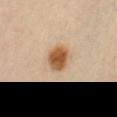Q: What did automated image analysis measure?
A: a footprint of about 7.5 mm² and a shape-asymmetry score of about 0.1 (0 = symmetric); an automated nevus-likeness rating near 100 out of 100 and lesion-presence confidence of about 100/100
Q: Patient demographics?
A: female, about 65 years old
Q: How was this image acquired?
A: ~15 mm tile from a whole-body skin photo
Q: What is the lesion's diameter?
A: about 3.5 mm
Q: How was the tile lit?
A: cross-polarized
Q: Lesion location?
A: the right thigh